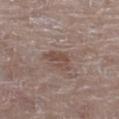Imaged during a routine full-body skin examination; the lesion was not biopsied and no histopathology is available. The lesion's longest dimension is about 3.5 mm. A female patient, approximately 85 years of age or older. A region of skin cropped from a whole-body photographic capture, roughly 15 mm wide. The lesion-visualizer software estimated a lesion–skin lightness drop of about 8 and a lesion-to-skin contrast of about 6.5 (normalized; higher = more distinct). And it measured internal color variation of about 1.5 on a 0–10 scale. The software also gave a classifier nevus-likeness of about 50/100 and lesion-presence confidence of about 100/100. The tile uses white-light illumination. The lesion is located on the left thigh.